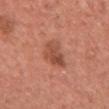Part of a total-body skin-imaging series; this lesion was reviewed on a skin check and was not flagged for biopsy.
On the chest.
The recorded lesion diameter is about 4 mm.
A female subject, approximately 40 years of age.
A 15 mm close-up extracted from a 3D total-body photography capture.
The tile uses white-light illumination.